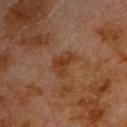An algorithmic analysis of the crop reported a lesion color around L≈28 a*≈18 b*≈27 in CIELAB, about 6 CIELAB-L* units darker than the surrounding skin, and a normalized lesion–skin contrast near 7.5. It also reported a border-irregularity rating of about 4.5/10 and internal color variation of about 2 on a 0–10 scale.
From the left upper arm.
A male patient, aged 78 to 82.
A 15 mm crop from a total-body photograph taken for skin-cancer surveillance.
Imaged with cross-polarized lighting.